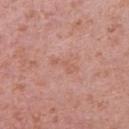<tbp_lesion>
<biopsy_status>not biopsied; imaged during a skin examination</biopsy_status>
<lighting>white-light</lighting>
<automated_metrics>
  <cielab_L>59</cielab_L>
  <cielab_a>23</cielab_a>
  <cielab_b>29</cielab_b>
  <vs_skin_darker_L>5.0</vs_skin_darker_L>
  <vs_skin_contrast_norm>4.5</vs_skin_contrast_norm>
  <nevus_likeness_0_100>0</nevus_likeness_0_100>
  <lesion_detection_confidence_0_100>100</lesion_detection_confidence_0_100>
</automated_metrics>
<patient>
  <sex>male</sex>
  <age_approx>40</age_approx>
</patient>
<site>right upper arm</site>
<lesion_size>
  <long_diameter_mm_approx>3.5</long_diameter_mm_approx>
</lesion_size>
<image>
  <source>total-body photography crop</source>
  <field_of_view_mm>15</field_of_view_mm>
</image>
</tbp_lesion>